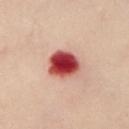Clinical summary: A region of skin cropped from a whole-body photographic capture, roughly 15 mm wide. An algorithmic analysis of the crop reported an area of roughly 11 mm², an eccentricity of roughly 0.55, and two-axis asymmetry of about 0.15. It also reported a lesion color around L≈41 a*≈33 b*≈25 in CIELAB, about 21 CIELAB-L* units darker than the surrounding skin, and a lesion-to-skin contrast of about 15 (normalized; higher = more distinct). It also reported a border-irregularity index near 1.5/10, a color-variation rating of about 7/10, and a peripheral color-asymmetry measure near 1.5. The lesion is located on the chest. The subject is a female roughly 60 years of age.